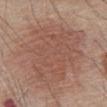biopsy status: catalogued during a skin exam; not biopsied | acquisition: total-body-photography crop, ~15 mm field of view | lighting: white-light illumination | subject: male, aged 78 to 82 | lesion diameter: about 11 mm | body site: the abdomen | automated lesion analysis: an area of roughly 55 mm², a shape eccentricity near 0.75, and a symmetry-axis asymmetry near 0.3; a lesion color around L≈52 a*≈20 b*≈26 in CIELAB; a border-irregularity rating of about 4/10, internal color variation of about 2.5 on a 0–10 scale, and a peripheral color-asymmetry measure near 1; a nevus-likeness score of about 0/100 and a lesion-detection confidence of about 95/100.